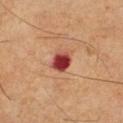This lesion was catalogued during total-body skin photography and was not selected for biopsy. About 2.5 mm across. The tile uses cross-polarized illumination. A roughly 15 mm field-of-view crop from a total-body skin photograph. From the left thigh. A male patient, approximately 60 years of age.